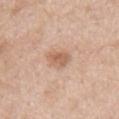Case summary:
- notes: catalogued during a skin exam; not biopsied
- lighting: white-light
- image source: total-body-photography crop, ~15 mm field of view
- patient: male, aged approximately 65
- site: the mid back
- automated lesion analysis: an area of roughly 5 mm², a shape eccentricity near 0.65, and a shape-asymmetry score of about 0.3 (0 = symmetric); border irregularity of about 2.5 on a 0–10 scale, a color-variation rating of about 2.5/10, and radial color variation of about 1